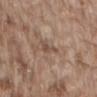Case summary:
– workup — no biopsy performed (imaged during a skin exam)
– subject — male, aged approximately 75
– acquisition — 15 mm crop, total-body photography
– site — the lower back